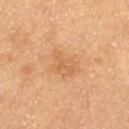{
  "biopsy_status": "not biopsied; imaged during a skin examination",
  "lesion_size": {
    "long_diameter_mm_approx": 4.0
  },
  "image": {
    "source": "total-body photography crop",
    "field_of_view_mm": 15
  },
  "patient": {
    "sex": "female",
    "age_approx": 55
  },
  "automated_metrics": {
    "area_mm2_approx": 8.0,
    "shape_asymmetry": 0.3,
    "border_irregularity_0_10": 3.5,
    "lesion_detection_confidence_0_100": 100
  },
  "site": "left thigh",
  "lighting": "cross-polarized"
}